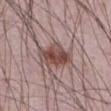Impression:
No biopsy was performed on this lesion — it was imaged during a full skin examination and was not determined to be concerning.
Acquisition and patient details:
The recorded lesion diameter is about 4.5 mm. This is a white-light tile. On the abdomen. A male patient approximately 65 years of age. A 15 mm close-up extracted from a 3D total-body photography capture.